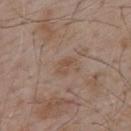This lesion was catalogued during total-body skin photography and was not selected for biopsy. On the upper back. Longest diameter approximately 2.5 mm. This is a white-light tile. The lesion-visualizer software estimated an outline eccentricity of about 0.8 (0 = round, 1 = elongated) and a symmetry-axis asymmetry near 0.35. The software also gave a border-irregularity rating of about 3.5/10, a within-lesion color-variation index near 2/10, and a peripheral color-asymmetry measure near 0.5. It also reported a lesion-detection confidence of about 100/100. A male patient, approximately 55 years of age. A 15 mm crop from a total-body photograph taken for skin-cancer surveillance.